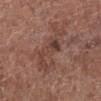This lesion was catalogued during total-body skin photography and was not selected for biopsy. This is a white-light tile. Located on the left lower leg. A lesion tile, about 15 mm wide, cut from a 3D total-body photograph. The patient is a female in their 80s. Measured at roughly 7 mm in maximum diameter. The lesion-visualizer software estimated a footprint of about 15 mm² and an eccentricity of roughly 0.95.